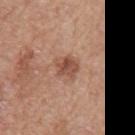- subject: female, aged 53 to 57
- size: ≈3 mm
- TBP lesion metrics: an area of roughly 5.5 mm² and a shape-asymmetry score of about 0.25 (0 = symmetric); an average lesion color of about L≈50 a*≈22 b*≈29 (CIELAB), roughly 11 lightness units darker than nearby skin, and a lesion-to-skin contrast of about 7.5 (normalized; higher = more distinct); a lesion-detection confidence of about 100/100
- imaging modality: total-body-photography crop, ~15 mm field of view
- site: the left upper arm